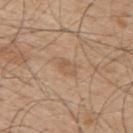  biopsy_status: not biopsied; imaged during a skin examination
  image:
    source: total-body photography crop
    field_of_view_mm: 15
  patient:
    sex: male
    age_approx: 50
  site: upper back